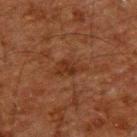Clinical impression:
Part of a total-body skin-imaging series; this lesion was reviewed on a skin check and was not flagged for biopsy.
Background:
A roughly 15 mm field-of-view crop from a total-body skin photograph. Located on the upper back. This is a cross-polarized tile. Approximately 3.5 mm at its widest. A male subject about 60 years old. The lesion-visualizer software estimated an outline eccentricity of about 0.8 (0 = round, 1 = elongated) and a symmetry-axis asymmetry near 0.35. And it measured lesion-presence confidence of about 100/100.